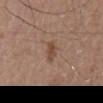notes: catalogued during a skin exam; not biopsied
lighting: white-light
acquisition: ~15 mm tile from a whole-body skin photo
patient: male, in their mid-70s
anatomic site: the back